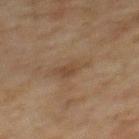Assessment:
The lesion was tiled from a total-body skin photograph and was not biopsied.
Acquisition and patient details:
The lesion is on the upper back. A female patient approximately 60 years of age. This image is a 15 mm lesion crop taken from a total-body photograph.